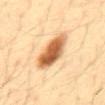Q: Was a biopsy performed?
A: catalogued during a skin exam; not biopsied
Q: What kind of image is this?
A: 15 mm crop, total-body photography
Q: What are the patient's age and sex?
A: male, aged 33 to 37
Q: Illumination type?
A: cross-polarized
Q: Lesion location?
A: the abdomen